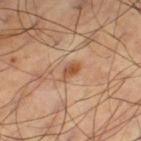{"image": {"source": "total-body photography crop", "field_of_view_mm": 15}, "lighting": "cross-polarized", "site": "left thigh", "patient": {"sex": "male", "age_approx": 60}, "automated_metrics": {"area_mm2_approx": 4.0, "eccentricity": 0.85, "shape_asymmetry": 0.3, "nevus_likeness_0_100": 75, "lesion_detection_confidence_0_100": 100}}